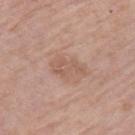<case>
<biopsy_status>not biopsied; imaged during a skin examination</biopsy_status>
<site>leg</site>
<patient>
  <sex>male</sex>
  <age_approx>75</age_approx>
</patient>
<lesion_size>
  <long_diameter_mm_approx>4.0</long_diameter_mm_approx>
</lesion_size>
<image>
  <source>total-body photography crop</source>
  <field_of_view_mm>15</field_of_view_mm>
</image>
<automated_metrics>
  <shape_asymmetry>0.4</shape_asymmetry>
</automated_metrics>
</case>